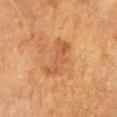Clinical impression:
Captured during whole-body skin photography for melanoma surveillance; the lesion was not biopsied.
Clinical summary:
A 15 mm close-up tile from a total-body photography series done for melanoma screening. Measured at roughly 5 mm in maximum diameter. From the right forearm. A female subject aged 53 to 57. Imaged with cross-polarized lighting.